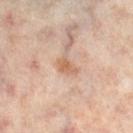workup — total-body-photography surveillance lesion; no biopsy | site — the right lower leg | acquisition — ~15 mm crop, total-body skin-cancer survey | patient — female, roughly 50 years of age | size — ≈2.5 mm | TBP lesion metrics — a lesion area of about 3 mm², a shape eccentricity near 0.8, and two-axis asymmetry of about 0.35; a normalized lesion–skin contrast near 7.5; a border-irregularity rating of about 3.5/10 and a within-lesion color-variation index near 1/10.